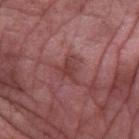{"biopsy_status": "not biopsied; imaged during a skin examination", "site": "left forearm", "image": {"source": "total-body photography crop", "field_of_view_mm": 15}, "lighting": "white-light", "patient": {"sex": "male", "age_approx": 55}, "lesion_size": {"long_diameter_mm_approx": 3.0}}